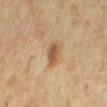notes = no biopsy performed (imaged during a skin exam) | image = total-body-photography crop, ~15 mm field of view | subject = female, about 65 years old | location = the back.